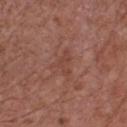  image:
    source: total-body photography crop
    field_of_view_mm: 15
  automated_metrics:
    area_mm2_approx: 5.0
    eccentricity: 0.75
    shape_asymmetry: 0.55
    border_irregularity_0_10: 6.0
    peripheral_color_asymmetry: 0.5
  patient:
    sex: male
    age_approx: 75
  lighting: white-light
  site: chest